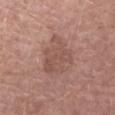Imaged during a routine full-body skin examination; the lesion was not biopsied and no histopathology is available.
The tile uses white-light illumination.
The lesion's longest dimension is about 5.5 mm.
Cropped from a whole-body photographic skin survey; the tile spans about 15 mm.
On the left forearm.
An algorithmic analysis of the crop reported a shape-asymmetry score of about 0.3 (0 = symmetric). It also reported a mean CIELAB color near L≈52 a*≈20 b*≈24 and a lesion–skin lightness drop of about 7.
A female subject, in their mid- to late 60s.